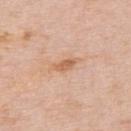follow-up — imaged on a skin check; not biopsied
patient — male, approximately 75 years of age
TBP lesion metrics — a mean CIELAB color near L≈63 a*≈22 b*≈35, a lesion–skin lightness drop of about 9, and a normalized lesion–skin contrast near 7; a border-irregularity index near 3/10 and peripheral color asymmetry of about 0.5
site — the upper back
image source — 15 mm crop, total-body photography